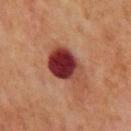Clinical impression: The lesion was photographed on a routine skin check and not biopsied; there is no pathology result. Image and clinical context: From the mid back. Approximately 5 mm at its widest. A roughly 15 mm field-of-view crop from a total-body skin photograph. The lesion-visualizer software estimated a footprint of about 14 mm², a shape eccentricity near 0.65, and a symmetry-axis asymmetry near 0.3. And it measured a lesion color around L≈33 a*≈28 b*≈24 in CIELAB and a normalized border contrast of about 15. The patient is a male aged 58–62.